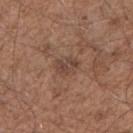{"biopsy_status": "not biopsied; imaged during a skin examination", "automated_metrics": {"cielab_L": 43, "cielab_a": 18, "cielab_b": 25, "vs_skin_darker_L": 8.0, "vs_skin_contrast_norm": 6.5, "nevus_likeness_0_100": 0}, "patient": {"sex": "male", "age_approx": 50}, "lesion_size": {"long_diameter_mm_approx": 2.5}, "lighting": "white-light", "image": {"source": "total-body photography crop", "field_of_view_mm": 15}, "site": "right upper arm"}